From the back; a 15 mm crop from a total-body photograph taken for skin-cancer surveillance; a male patient, approximately 40 years of age: 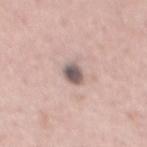Histopathologically confirmed as a dysplastic (Clark) nevus.